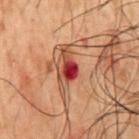subject — male, about 50 years old | imaging modality — 15 mm crop, total-body photography | size — about 2.5 mm | image-analysis metrics — a lesion–skin lightness drop of about 15 and a normalized border contrast of about 13; a border-irregularity index near 3/10, internal color variation of about 5 on a 0–10 scale, and a peripheral color-asymmetry measure near 2 | body site — the mid back.